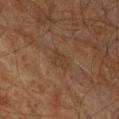<case>
  <biopsy_status>not biopsied; imaged during a skin examination</biopsy_status>
  <image>
    <source>total-body photography crop</source>
    <field_of_view_mm>15</field_of_view_mm>
  </image>
  <site>chest</site>
  <patient>
    <sex>male</sex>
    <age_approx>60</age_approx>
  </patient>
</case>